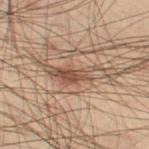{
  "biopsy_status": "not biopsied; imaged during a skin examination",
  "patient": {
    "sex": "male",
    "age_approx": 55
  },
  "lesion_size": {
    "long_diameter_mm_approx": 6.0
  },
  "image": {
    "source": "total-body photography crop",
    "field_of_view_mm": 15
  },
  "site": "left thigh"
}